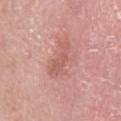The lesion was tiled from a total-body skin photograph and was not biopsied. Imaged with white-light lighting. The subject is a male aged 48–52. The lesion is located on the leg. A 15 mm close-up tile from a total-body photography series done for melanoma screening.